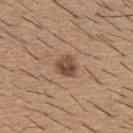workup = imaged on a skin check; not biopsied
lighting = white-light illumination
anatomic site = the upper back
lesion diameter = about 2.5 mm
acquisition = 15 mm crop, total-body photography
patient = male, roughly 60 years of age